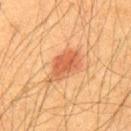follow-up: no biopsy performed (imaged during a skin exam); patient: male, about 35 years old; tile lighting: cross-polarized; lesion size: ≈5.5 mm; anatomic site: the upper back; image: ~15 mm crop, total-body skin-cancer survey.